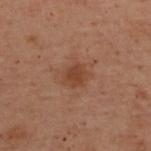Imaged during a routine full-body skin examination; the lesion was not biopsied and no histopathology is available.
A female patient aged 53–57.
A close-up tile cropped from a whole-body skin photograph, about 15 mm across.
The tile uses cross-polarized illumination.
Located on the back.
The lesion-visualizer software estimated a footprint of about 5.5 mm² and a shape-asymmetry score of about 0.15 (0 = symmetric). The software also gave an average lesion color of about L≈35 a*≈19 b*≈26 (CIELAB), a lesion–skin lightness drop of about 7, and a normalized lesion–skin contrast near 6.5. The software also gave a border-irregularity index near 1.5/10, a color-variation rating of about 1.5/10, and a peripheral color-asymmetry measure near 0.5. The analysis additionally found a classifier nevus-likeness of about 25/100 and lesion-presence confidence of about 100/100.
Measured at roughly 3 mm in maximum diameter.